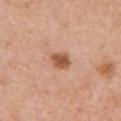The lesion was photographed on a routine skin check and not biopsied; there is no pathology result.
Located on the chest.
Longest diameter approximately 2.5 mm.
This is a white-light tile.
The total-body-photography lesion software estimated a border-irregularity rating of about 1.5/10, a color-variation rating of about 2.5/10, and peripheral color asymmetry of about 0.5. The analysis additionally found lesion-presence confidence of about 100/100.
A 15 mm close-up tile from a total-body photography series done for melanoma screening.
A female patient in their mid-40s.